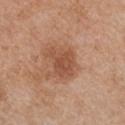Assessment: Recorded during total-body skin imaging; not selected for excision or biopsy. Acquisition and patient details: Imaged with white-light lighting. The subject is a female about 40 years old. This image is a 15 mm lesion crop taken from a total-body photograph. The lesion is located on the chest. Longest diameter approximately 4.5 mm.